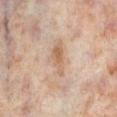biopsy_status: not biopsied; imaged during a skin examination
image:
  source: total-body photography crop
  field_of_view_mm: 15
site: right lower leg
automated_metrics:
  area_mm2_approx: 6.0
  eccentricity: 0.9
  shape_asymmetry: 0.35
  vs_skin_darker_L: 9.0
patient:
  sex: female
  age_approx: 50